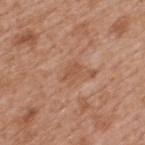follow-up: total-body-photography surveillance lesion; no biopsy | patient: male, roughly 65 years of age | acquisition: ~15 mm crop, total-body skin-cancer survey | image-analysis metrics: an automated nevus-likeness rating near 0 out of 100 and a detector confidence of about 100 out of 100 that the crop contains a lesion | anatomic site: the upper back.